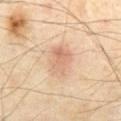Image and clinical context:
A roughly 15 mm field-of-view crop from a total-body skin photograph. Located on the front of the torso. Approximately 4 mm at its widest. The total-body-photography lesion software estimated a footprint of about 10 mm², an eccentricity of roughly 0.65, and two-axis asymmetry of about 0.2. And it measured a mean CIELAB color near L≈67 a*≈19 b*≈32 and about 9 CIELAB-L* units darker than the surrounding skin. It also reported a border-irregularity rating of about 2/10 and internal color variation of about 5.5 on a 0–10 scale. The patient is a male approximately 70 years of age. Imaged with cross-polarized lighting.